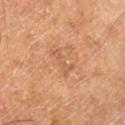  biopsy_status: not biopsied; imaged during a skin examination
  patient:
    sex: male
    age_approx: 65
  automated_metrics:
    vs_skin_darker_L: 7.0
    vs_skin_contrast_norm: 4.5
    border_irregularity_0_10: 7.5
    peripheral_color_asymmetry: 0.0
  lighting: cross-polarized
  lesion_size:
    long_diameter_mm_approx: 3.5
  image:
    source: total-body photography crop
    field_of_view_mm: 15
  site: leg